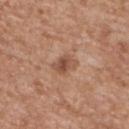Notes:
* subject: male, approximately 65 years of age
* anatomic site: the back
* imaging modality: 15 mm crop, total-body photography
* tile lighting: white-light
* TBP lesion metrics: a border-irregularity rating of about 2.5/10 and a peripheral color-asymmetry measure near 1.5; a nevus-likeness score of about 80/100 and a lesion-detection confidence of about 100/100